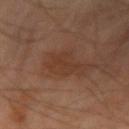The lesion was photographed on a routine skin check and not biopsied; there is no pathology result. The patient is a male approximately 70 years of age. The lesion is on the left forearm. Imaged with cross-polarized lighting. The lesion-visualizer software estimated an average lesion color of about L≈35 a*≈19 b*≈28 (CIELAB), roughly 5 lightness units darker than nearby skin, and a normalized lesion–skin contrast near 5. It also reported a border-irregularity rating of about 5/10 and radial color variation of about 0.5. Approximately 3.5 mm at its widest. A 15 mm close-up extracted from a 3D total-body photography capture.